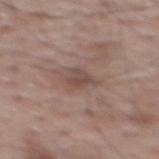Clinical impression: No biopsy was performed on this lesion — it was imaged during a full skin examination and was not determined to be concerning. Context: Captured under white-light illumination. This image is a 15 mm lesion crop taken from a total-body photograph. The lesion's longest dimension is about 3 mm. The subject is a male aged around 70. From the back.